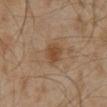The lesion is located on the abdomen. Automated tile analysis of the lesion measured a footprint of about 6 mm², an eccentricity of roughly 0.5, and a shape-asymmetry score of about 0.2 (0 = symmetric). It also reported a border-irregularity rating of about 1.5/10, a within-lesion color-variation index near 3/10, and peripheral color asymmetry of about 1. The analysis additionally found an automated nevus-likeness rating near 55 out of 100 and a lesion-detection confidence of about 100/100. A male subject in their 60s. This is a cross-polarized tile. A 15 mm crop from a total-body photograph taken for skin-cancer surveillance.